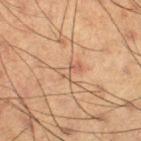No biopsy was performed on this lesion — it was imaged during a full skin examination and was not determined to be concerning. The subject is a male aged 58 to 62. Automated image analysis of the tile measured a lesion area of about 2.5 mm², a shape eccentricity near 0.9, and two-axis asymmetry of about 0.5. And it measured a lesion color around L≈45 a*≈17 b*≈26 in CIELAB, a lesion–skin lightness drop of about 6, and a normalized border contrast of about 5. It also reported a classifier nevus-likeness of about 0/100 and lesion-presence confidence of about 100/100. Imaged with cross-polarized lighting. Longest diameter approximately 2.5 mm. Located on the leg. A lesion tile, about 15 mm wide, cut from a 3D total-body photograph.